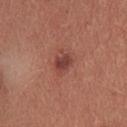notes=imaged on a skin check; not biopsied
subject=female, approximately 35 years of age
automated metrics=a lesion area of about 4 mm² and a shape eccentricity near 0.7; a mean CIELAB color near L≈42 a*≈27 b*≈25, a lesion–skin lightness drop of about 10, and a normalized lesion–skin contrast near 8; border irregularity of about 3 on a 0–10 scale, a within-lesion color-variation index near 3.5/10, and peripheral color asymmetry of about 1; lesion-presence confidence of about 100/100
location=the head or neck
size=about 2.5 mm
acquisition=~15 mm crop, total-body skin-cancer survey